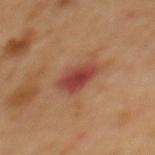The lesion was photographed on a routine skin check and not biopsied; there is no pathology result.
A male patient aged 63–67.
Measured at roughly 4 mm in maximum diameter.
The lesion is on the mid back.
Automated image analysis of the tile measured a lesion color around L≈44 a*≈27 b*≈29 in CIELAB and a normalized border contrast of about 9. The analysis additionally found an automated nevus-likeness rating near 5 out of 100 and a lesion-detection confidence of about 100/100.
A 15 mm close-up extracted from a 3D total-body photography capture.
The tile uses cross-polarized illumination.